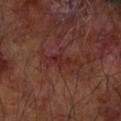<case>
  <biopsy_status>not biopsied; imaged during a skin examination</biopsy_status>
  <site>left forearm</site>
  <lesion_size>
    <long_diameter_mm_approx>3.0</long_diameter_mm_approx>
  </lesion_size>
  <lighting>cross-polarized</lighting>
  <patient>
    <sex>male</sex>
    <age_approx>65</age_approx>
  </patient>
  <image>
    <source>total-body photography crop</source>
    <field_of_view_mm>15</field_of_view_mm>
  </image>
</case>